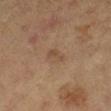Notes:
* lesion size — ~2.5 mm (longest diameter)
* acquisition — ~15 mm crop, total-body skin-cancer survey
* tile lighting — cross-polarized illumination
* patient — female, aged 58 to 62
* location — the right lower leg